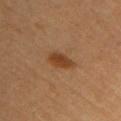Clinical impression:
The lesion was photographed on a routine skin check and not biopsied; there is no pathology result.
Context:
Longest diameter approximately 3.5 mm. The patient is a female approximately 60 years of age. A close-up tile cropped from a whole-body skin photograph, about 15 mm across. The lesion is on the right upper arm. This is a cross-polarized tile.